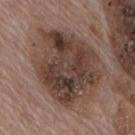The lesion was photographed on a routine skin check and not biopsied; there is no pathology result.